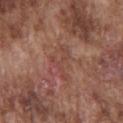A male patient aged approximately 75. Cropped from a total-body skin-imaging series; the visible field is about 15 mm. About 4.5 mm across. Captured under white-light illumination. On the front of the torso.